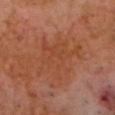Assessment:
Recorded during total-body skin imaging; not selected for excision or biopsy.
Background:
A male patient, approximately 60 years of age. Cropped from a whole-body photographic skin survey; the tile spans about 15 mm. From the head or neck.